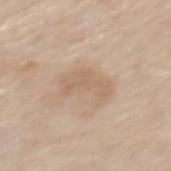Impression:
Imaged during a routine full-body skin examination; the lesion was not biopsied and no histopathology is available.
Clinical summary:
A 15 mm close-up extracted from a 3D total-body photography capture. Located on the mid back. The lesion's longest dimension is about 5 mm. A male patient, aged 63–67. Imaged with white-light lighting.